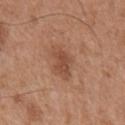Case summary:
* biopsy status: no biopsy performed (imaged during a skin exam)